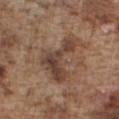follow-up = imaged on a skin check; not biopsied
TBP lesion metrics = a mean CIELAB color near L≈43 a*≈17 b*≈26, about 10 CIELAB-L* units darker than the surrounding skin, and a normalized border contrast of about 8; border irregularity of about 8.5 on a 0–10 scale and peripheral color asymmetry of about 1.5
subject = male, aged 73 to 77
acquisition = ~15 mm tile from a whole-body skin photo
size = about 6.5 mm
site = the chest